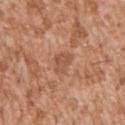Q: Is there a histopathology result?
A: no biopsy performed (imaged during a skin exam)
Q: Patient demographics?
A: male, in their mid- to late 40s
Q: How large is the lesion?
A: ≈2.5 mm
Q: Lesion location?
A: the arm
Q: What did automated image analysis measure?
A: about 8 CIELAB-L* units darker than the surrounding skin; a border-irregularity rating of about 3.5/10 and peripheral color asymmetry of about 1
Q: How was this image acquired?
A: total-body-photography crop, ~15 mm field of view